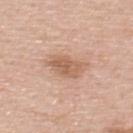Clinical impression: The lesion was photographed on a routine skin check and not biopsied; there is no pathology result. Background: The total-body-photography lesion software estimated a mean CIELAB color near L≈60 a*≈20 b*≈31 and about 10 CIELAB-L* units darker than the surrounding skin. And it measured a classifier nevus-likeness of about 20/100. The lesion is on the upper back. A male patient aged approximately 50. Measured at roughly 4 mm in maximum diameter. This image is a 15 mm lesion crop taken from a total-body photograph.